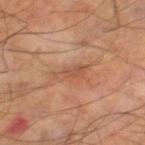Captured during whole-body skin photography for melanoma surveillance; the lesion was not biopsied. About 4 mm across. Captured under cross-polarized illumination. The subject is a male roughly 55 years of age. Automated tile analysis of the lesion measured a shape eccentricity near 0.9 and two-axis asymmetry of about 0.35. The analysis additionally found a nevus-likeness score of about 0/100 and a detector confidence of about 100 out of 100 that the crop contains a lesion. On the right thigh. A 15 mm crop from a total-body photograph taken for skin-cancer surveillance.